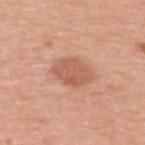No biopsy was performed on this lesion — it was imaged during a full skin examination and was not determined to be concerning. Located on the upper back. A male patient about 45 years old. The lesion's longest dimension is about 4 mm. This is a white-light tile. A region of skin cropped from a whole-body photographic capture, roughly 15 mm wide.